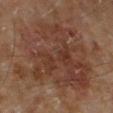| feature | finding |
|---|---|
| follow-up | total-body-photography surveillance lesion; no biopsy |
| subject | male, aged approximately 60 |
| automated lesion analysis | border irregularity of about 7 on a 0–10 scale, a color-variation rating of about 5/10, and radial color variation of about 1.5; an automated nevus-likeness rating near 5 out of 100 and a lesion-detection confidence of about 100/100 |
| image | ~15 mm crop, total-body skin-cancer survey |
| lighting | cross-polarized |
| location | the leg |
| lesion diameter | ≈11 mm |